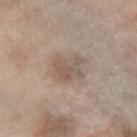follow-up: imaged on a skin check; not biopsied | illumination: white-light | image-analysis metrics: a classifier nevus-likeness of about 70/100 | lesion diameter: about 4 mm | body site: the right forearm | image source: total-body-photography crop, ~15 mm field of view | subject: female, in their 70s.